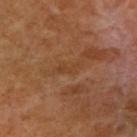Q: Is there a histopathology result?
A: no biopsy performed (imaged during a skin exam)
Q: What kind of image is this?
A: 15 mm crop, total-body photography
Q: Automated lesion metrics?
A: a shape eccentricity near 0.95 and a shape-asymmetry score of about 0.45 (0 = symmetric); an average lesion color of about L≈42 a*≈21 b*≈35 (CIELAB) and a normalized lesion–skin contrast near 5; border irregularity of about 5 on a 0–10 scale and a within-lesion color-variation index near 0/10; a classifier nevus-likeness of about 0/100 and lesion-presence confidence of about 75/100
Q: How was the tile lit?
A: cross-polarized
Q: Who is the patient?
A: female, in their mid-50s
Q: Where on the body is the lesion?
A: the right upper arm
Q: How large is the lesion?
A: about 3 mm